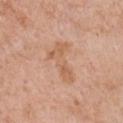Case summary:
– acquisition · total-body-photography crop, ~15 mm field of view
– patient · female, aged 38 to 42
– lighting · white-light
– anatomic site · the chest
– size · ~5 mm (longest diameter)